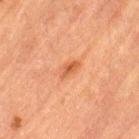{
  "biopsy_status": "not biopsied; imaged during a skin examination",
  "site": "left thigh",
  "image": {
    "source": "total-body photography crop",
    "field_of_view_mm": 15
  },
  "patient": {
    "sex": "male",
    "age_approx": "85+"
  },
  "automated_metrics": {
    "area_mm2_approx": 3.0,
    "eccentricity": 0.85,
    "cielab_L": 51,
    "cielab_a": 27,
    "cielab_b": 37,
    "vs_skin_darker_L": 9.0,
    "vs_skin_contrast_norm": 7.0,
    "nevus_likeness_0_100": 60,
    "lesion_detection_confidence_0_100": 100
  }
}